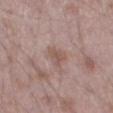No biopsy was performed on this lesion — it was imaged during a full skin examination and was not determined to be concerning. The recorded lesion diameter is about 3 mm. Cropped from a whole-body photographic skin survey; the tile spans about 15 mm. Imaged with white-light lighting. A male subject, aged 48–52. An algorithmic analysis of the crop reported a border-irregularity index near 3.5/10 and radial color variation of about 0.5. It also reported an automated nevus-likeness rating near 0 out of 100 and a detector confidence of about 100 out of 100 that the crop contains a lesion. From the left thigh.